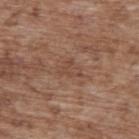The lesion was photographed on a routine skin check and not biopsied; there is no pathology result.
A lesion tile, about 15 mm wide, cut from a 3D total-body photograph.
Automated image analysis of the tile measured border irregularity of about 4.5 on a 0–10 scale and radial color variation of about 1.
The tile uses white-light illumination.
The patient is a male approximately 70 years of age.
Measured at roughly 3 mm in maximum diameter.
From the upper back.